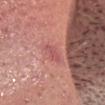The subject is a male about 60 years old. A 15 mm close-up tile from a total-body photography series done for melanoma screening. An algorithmic analysis of the crop reported a lesion color around L≈54 a*≈31 b*≈23 in CIELAB and a lesion-to-skin contrast of about 5 (normalized; higher = more distinct). And it measured border irregularity of about 3.5 on a 0–10 scale, internal color variation of about 1 on a 0–10 scale, and radial color variation of about 0. The analysis additionally found a nevus-likeness score of about 0/100 and lesion-presence confidence of about 100/100. Longest diameter approximately 2 mm. On the head or neck.